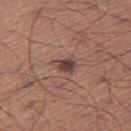Q: Was this lesion biopsied?
A: imaged on a skin check; not biopsied
Q: How was this image acquired?
A: 15 mm crop, total-body photography
Q: How large is the lesion?
A: about 3 mm
Q: Who is the patient?
A: male, aged approximately 65
Q: What lighting was used for the tile?
A: white-light illumination
Q: Where on the body is the lesion?
A: the right thigh